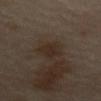Clinical summary:
This is a cross-polarized tile. A 15 mm close-up tile from a total-body photography series done for melanoma screening. The lesion's longest dimension is about 2.5 mm. The lesion is on the abdomen. A female subject aged around 50.